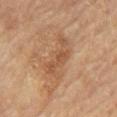Recorded during total-body skin imaging; not selected for excision or biopsy. A male patient aged approximately 70. Automated image analysis of the tile measured a footprint of about 11 mm² and two-axis asymmetry of about 0.5. The analysis additionally found an average lesion color of about L≈52 a*≈20 b*≈34 (CIELAB), roughly 8 lightness units darker than nearby skin, and a lesion-to-skin contrast of about 6 (normalized; higher = more distinct). It also reported a nevus-likeness score of about 0/100 and a detector confidence of about 100 out of 100 that the crop contains a lesion. This is a cross-polarized tile. Measured at roughly 6 mm in maximum diameter. Cropped from a whole-body photographic skin survey; the tile spans about 15 mm. Located on the chest.